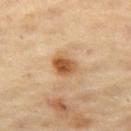Part of a total-body skin-imaging series; this lesion was reviewed on a skin check and was not flagged for biopsy. Captured under cross-polarized illumination. The lesion is on the left thigh. Measured at roughly 3.5 mm in maximum diameter. The patient is a female aged approximately 70. A close-up tile cropped from a whole-body skin photograph, about 15 mm across.